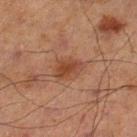Clinical summary: Measured at roughly 3.5 mm in maximum diameter. A male patient roughly 70 years of age. The lesion is on the left lower leg. A lesion tile, about 15 mm wide, cut from a 3D total-body photograph. Captured under cross-polarized illumination.